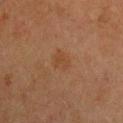diameter=about 2.5 mm | subject=female, aged 48 to 52 | tile lighting=cross-polarized illumination | image=total-body-photography crop, ~15 mm field of view | site=the front of the torso.